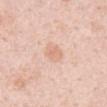follow-up: no biopsy performed (imaged during a skin exam) | lesion size: about 2.5 mm | image source: ~15 mm tile from a whole-body skin photo | site: the abdomen | illumination: white-light illumination | automated lesion analysis: a mean CIELAB color near L≈72 a*≈21 b*≈30, a lesion–skin lightness drop of about 7, and a lesion-to-skin contrast of about 4.5 (normalized; higher = more distinct); a border-irregularity rating of about 2.5/10, a color-variation rating of about 2.5/10, and radial color variation of about 1; a nevus-likeness score of about 5/100 and lesion-presence confidence of about 100/100 | patient: male, roughly 50 years of age.